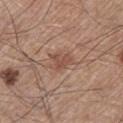The lesion was tiled from a total-body skin photograph and was not biopsied.
A male patient roughly 65 years of age.
Cropped from a total-body skin-imaging series; the visible field is about 15 mm.
Located on the left thigh.
Imaged with white-light lighting.
Longest diameter approximately 3.5 mm.